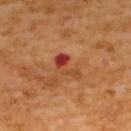Cropped from a whole-body photographic skin survey; the tile spans about 15 mm. The subject is a male about 60 years old. The lesion is located on the upper back. The total-body-photography lesion software estimated an area of roughly 5 mm², a shape eccentricity near 0.85, and two-axis asymmetry of about 0.55. The recorded lesion diameter is about 3.5 mm. Captured under cross-polarized illumination.